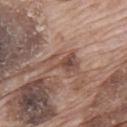Imaged during a routine full-body skin examination; the lesion was not biopsied and no histopathology is available.
The lesion is located on the upper back.
A lesion tile, about 15 mm wide, cut from a 3D total-body photograph.
The recorded lesion diameter is about 5 mm.
The tile uses white-light illumination.
The patient is a male roughly 70 years of age.